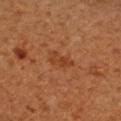  biopsy_status: not biopsied; imaged during a skin examination
  patient:
    sex: female
  image:
    source: total-body photography crop
    field_of_view_mm: 15
  site: right upper arm
  lighting: cross-polarized